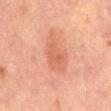Captured during whole-body skin photography for melanoma surveillance; the lesion was not biopsied.
A roughly 15 mm field-of-view crop from a total-body skin photograph.
A male subject aged around 65.
From the abdomen.